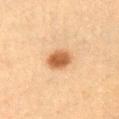<record>
  <biopsy_status>not biopsied; imaged during a skin examination</biopsy_status>
  <image>
    <source>total-body photography crop</source>
    <field_of_view_mm>15</field_of_view_mm>
  </image>
  <site>right upper arm</site>
  <patient>
    <sex>male</sex>
    <age_approx>55</age_approx>
  </patient>
  <lesion_size>
    <long_diameter_mm_approx>3.5</long_diameter_mm_approx>
  </lesion_size>
  <automated_metrics>
    <area_mm2_approx>7.0</area_mm2_approx>
    <eccentricity>0.55</eccentricity>
    <shape_asymmetry>0.2</shape_asymmetry>
    <cielab_L>52</cielab_L>
    <cielab_a>21</cielab_a>
    <cielab_b>36</cielab_b>
    <vs_skin_darker_L>14.0</vs_skin_darker_L>
    <vs_skin_contrast_norm>9.5</vs_skin_contrast_norm>
    <nevus_likeness_0_100>100</nevus_likeness_0_100>
    <lesion_detection_confidence_0_100>100</lesion_detection_confidence_0_100>
  </automated_metrics>
  <lighting>cross-polarized</lighting>
</record>